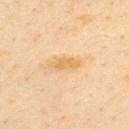Case summary:
– follow-up · catalogued during a skin exam; not biopsied
– patient · male, roughly 35 years of age
– image source · ~15 mm tile from a whole-body skin photo
– size · ~3.5 mm (longest diameter)
– body site · the back